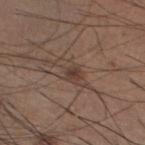<case>
  <patient>
    <sex>male</sex>
    <age_approx>35</age_approx>
  </patient>
  <lesion_size>
    <long_diameter_mm_approx>4.0</long_diameter_mm_approx>
  </lesion_size>
  <image>
    <source>total-body photography crop</source>
    <field_of_view_mm>15</field_of_view_mm>
  </image>
  <automated_metrics>
    <color_variation_0_10>4.0</color_variation_0_10>
    <peripheral_color_asymmetry>1.0</peripheral_color_asymmetry>
    <nevus_likeness_0_100>55</nevus_likeness_0_100>
  </automated_metrics>
  <lighting>white-light</lighting>
  <site>left lower leg</site>
</case>